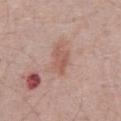This lesion was catalogued during total-body skin photography and was not selected for biopsy.